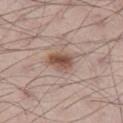No biopsy was performed on this lesion — it was imaged during a full skin examination and was not determined to be concerning. Cropped from a whole-body photographic skin survey; the tile spans about 15 mm. From the left thigh. Captured under white-light illumination. Automated image analysis of the tile measured an eccentricity of roughly 0.8 and a symmetry-axis asymmetry near 0.25. The software also gave a lesion color around L≈50 a*≈19 b*≈26 in CIELAB, roughly 13 lightness units darker than nearby skin, and a lesion-to-skin contrast of about 9.5 (normalized; higher = more distinct). And it measured border irregularity of about 2.5 on a 0–10 scale, a within-lesion color-variation index near 4/10, and peripheral color asymmetry of about 1.5. Approximately 3.5 mm at its widest. A male patient, about 70 years old.